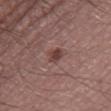biopsy_status: not biopsied; imaged during a skin examination
lighting: white-light
site: right thigh
image:
  source: total-body photography crop
  field_of_view_mm: 15
lesion_size:
  long_diameter_mm_approx: 2.5
automated_metrics:
  area_mm2_approx: 4.0
  eccentricity: 0.65
  shape_asymmetry: 0.35
  border_irregularity_0_10: 3.0
  color_variation_0_10: 2.5
  nevus_likeness_0_100: 75
  lesion_detection_confidence_0_100: 100
patient:
  sex: male
  age_approx: 50